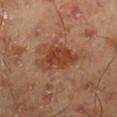Clinical impression: Recorded during total-body skin imaging; not selected for excision or biopsy. Context: A male patient aged approximately 45. This image is a 15 mm lesion crop taken from a total-body photograph. The lesion is located on the right lower leg.